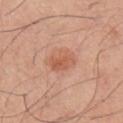Case summary:
– biopsy status — total-body-photography surveillance lesion; no biopsy
– location — the chest
– lesion size — ≈3.5 mm
– acquisition — 15 mm crop, total-body photography
– patient — male, in their mid-40s
– lighting — white-light illumination